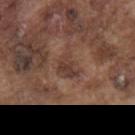<record>
  <biopsy_status>not biopsied; imaged during a skin examination</biopsy_status>
  <patient>
    <sex>male</sex>
    <age_approx>75</age_approx>
  </patient>
  <lighting>white-light</lighting>
  <lesion_size>
    <long_diameter_mm_approx>2.5</long_diameter_mm_approx>
  </lesion_size>
  <automated_metrics>
    <area_mm2_approx>4.5</area_mm2_approx>
    <eccentricity>0.5</eccentricity>
    <shape_asymmetry>0.45</shape_asymmetry>
  </automated_metrics>
  <image>
    <source>total-body photography crop</source>
    <field_of_view_mm>15</field_of_view_mm>
  </image>
  <site>mid back</site>
</record>